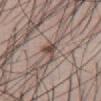The lesion was tiled from a total-body skin photograph and was not biopsied. The lesion is on the abdomen. A male subject aged 73 to 77. Cropped from a whole-body photographic skin survey; the tile spans about 15 mm.